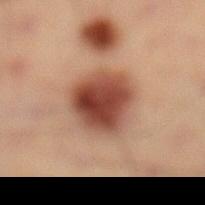Part of a total-body skin-imaging series; this lesion was reviewed on a skin check and was not flagged for biopsy. Located on the left lower leg. Cropped from a whole-body photographic skin survey; the tile spans about 15 mm. Longest diameter approximately 5 mm. This is a cross-polarized tile. A male subject, aged around 40.